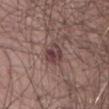Case summary:
* subject — male, in their mid- to late 70s
* location — the abdomen
* lesion diameter — ~3 mm (longest diameter)
* image source — total-body-photography crop, ~15 mm field of view
* lighting — white-light illumination